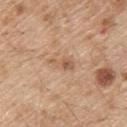notes: imaged on a skin check; not biopsied
anatomic site: the upper back
subject: male, roughly 70 years of age
lighting: white-light
lesion size: about 3.5 mm
image source: ~15 mm crop, total-body skin-cancer survey